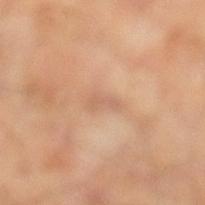The lesion was tiled from a total-body skin photograph and was not biopsied.
The lesion is on the left lower leg.
A lesion tile, about 15 mm wide, cut from a 3D total-body photograph.
Measured at roughly 2.5 mm in maximum diameter.
The tile uses cross-polarized illumination.
The subject is in their mid-50s.